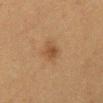Clinical impression: Imaged during a routine full-body skin examination; the lesion was not biopsied and no histopathology is available. Clinical summary: This image is a 15 mm lesion crop taken from a total-body photograph. The subject is a female aged approximately 50. The lesion is on the abdomen. Longest diameter approximately 3 mm.